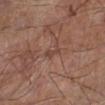Clinical impression:
The lesion was tiled from a total-body skin photograph and was not biopsied.
Context:
The patient is a male roughly 70 years of age. Located on the left lower leg. Cropped from a whole-body photographic skin survey; the tile spans about 15 mm.